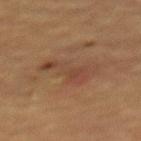Notes:
– biopsy status — total-body-photography surveillance lesion; no biopsy